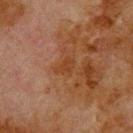* notes: catalogued during a skin exam; not biopsied
* imaging modality: ~15 mm tile from a whole-body skin photo
* subject: male, aged 78 to 82
* site: the upper back
* illumination: cross-polarized
* diameter: about 2.5 mm